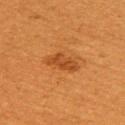| feature | finding |
|---|---|
| biopsy status | total-body-photography surveillance lesion; no biopsy |
| patient | female, aged approximately 55 |
| tile lighting | cross-polarized illumination |
| image source | total-body-photography crop, ~15 mm field of view |
| automated lesion analysis | an area of roughly 6.5 mm² and a symmetry-axis asymmetry near 0.3; border irregularity of about 3 on a 0–10 scale, a color-variation rating of about 2.5/10, and peripheral color asymmetry of about 1; an automated nevus-likeness rating near 90 out of 100 and a detector confidence of about 100 out of 100 that the crop contains a lesion |
| site | the right upper arm |
| lesion size | ≈4 mm |